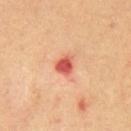Notes:
- imaging modality · ~15 mm crop, total-body skin-cancer survey
- size · ~2.5 mm (longest diameter)
- subject · male, aged 68–72
- anatomic site · the chest
- lighting · cross-polarized